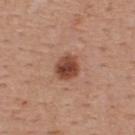Assessment:
The lesion was tiled from a total-body skin photograph and was not biopsied.
Background:
The patient is a male aged 53–57. Approximately 3 mm at its widest. This is a white-light tile. This image is a 15 mm lesion crop taken from a total-body photograph. Located on the upper back.